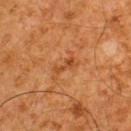This lesion was catalogued during total-body skin photography and was not selected for biopsy.
Longest diameter approximately 2.5 mm.
Automated image analysis of the tile measured a lesion area of about 2.5 mm², a shape eccentricity near 0.95, and a shape-asymmetry score of about 0.45 (0 = symmetric). And it measured a lesion color around L≈39 a*≈24 b*≈36 in CIELAB, about 8 CIELAB-L* units darker than the surrounding skin, and a normalized lesion–skin contrast near 6.5. The analysis additionally found a color-variation rating of about 0/10 and peripheral color asymmetry of about 0. And it measured a classifier nevus-likeness of about 0/100.
A male subject approximately 60 years of age.
A 15 mm close-up tile from a total-body photography series done for melanoma screening.
Imaged with cross-polarized lighting.
The lesion is located on the upper back.